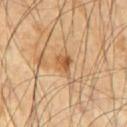Recorded during total-body skin imaging; not selected for excision or biopsy.
Captured under cross-polarized illumination.
An algorithmic analysis of the crop reported two-axis asymmetry of about 0.35. The software also gave a lesion color around L≈55 a*≈21 b*≈39 in CIELAB, roughly 10 lightness units darker than nearby skin, and a normalized border contrast of about 7.5. The analysis additionally found border irregularity of about 3 on a 0–10 scale and internal color variation of about 4.5 on a 0–10 scale. The software also gave a classifier nevus-likeness of about 45/100 and a detector confidence of about 100 out of 100 that the crop contains a lesion.
Approximately 2.5 mm at its widest.
A close-up tile cropped from a whole-body skin photograph, about 15 mm across.
The lesion is on the right upper arm.
A male patient aged around 60.